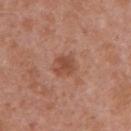Q: Was this lesion biopsied?
A: catalogued during a skin exam; not biopsied
Q: How was the tile lit?
A: white-light
Q: Lesion location?
A: the left upper arm
Q: How was this image acquired?
A: ~15 mm crop, total-body skin-cancer survey
Q: Who is the patient?
A: female, in their 40s